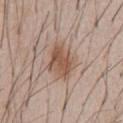Cropped from a total-body skin-imaging series; the visible field is about 15 mm. A male patient, in their mid- to late 50s. The total-body-photography lesion software estimated a footprint of about 12 mm² and an outline eccentricity of about 0.7 (0 = round, 1 = elongated). It also reported a peripheral color-asymmetry measure near 1.5. It also reported an automated nevus-likeness rating near 90 out of 100 and lesion-presence confidence of about 100/100. The lesion's longest dimension is about 5 mm.